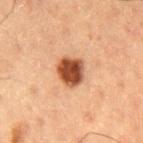Q: Was a biopsy performed?
A: imaged on a skin check; not biopsied
Q: What did automated image analysis measure?
A: an average lesion color of about L≈44 a*≈25 b*≈33 (CIELAB), about 19 CIELAB-L* units darker than the surrounding skin, and a lesion-to-skin contrast of about 13 (normalized; higher = more distinct); a within-lesion color-variation index near 5/10 and peripheral color asymmetry of about 1.5; an automated nevus-likeness rating near 100 out of 100 and lesion-presence confidence of about 100/100
Q: How was this image acquired?
A: ~15 mm tile from a whole-body skin photo
Q: Lesion size?
A: ≈3.5 mm
Q: Patient demographics?
A: male, aged 58–62